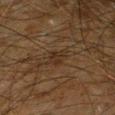biopsy status: no biopsy performed (imaged during a skin exam)
body site: the left forearm
automated metrics: a shape eccentricity near 0.25 and a symmetry-axis asymmetry near 0.3; a lesion color around L≈23 a*≈12 b*≈22 in CIELAB, a lesion–skin lightness drop of about 4, and a lesion-to-skin contrast of about 5.5 (normalized; higher = more distinct); a border-irregularity rating of about 3/10 and a color-variation rating of about 3.5/10; a classifier nevus-likeness of about 0/100 and a detector confidence of about 50 out of 100 that the crop contains a lesion
subject: male, approximately 65 years of age
illumination: cross-polarized illumination
image: ~15 mm tile from a whole-body skin photo